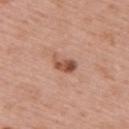Recorded during total-body skin imaging; not selected for excision or biopsy.
The lesion's longest dimension is about 3.5 mm.
A male patient, about 60 years old.
A region of skin cropped from a whole-body photographic capture, roughly 15 mm wide.
The lesion is located on the upper back.
The tile uses white-light illumination.